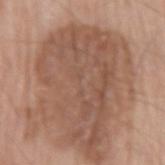Clinical impression: This lesion was catalogued during total-body skin photography and was not selected for biopsy. Image and clinical context: A 15 mm close-up tile from a total-body photography series done for melanoma screening. The patient is a male roughly 75 years of age. The lesion is on the left upper arm.